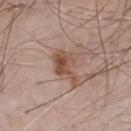Part of a total-body skin-imaging series; this lesion was reviewed on a skin check and was not flagged for biopsy. A region of skin cropped from a whole-body photographic capture, roughly 15 mm wide. Longest diameter approximately 4.5 mm. A male subject, aged 53–57. The total-body-photography lesion software estimated a footprint of about 7.5 mm², a shape eccentricity near 0.85, and two-axis asymmetry of about 0.45. It also reported a classifier nevus-likeness of about 65/100. The tile uses white-light illumination. The lesion is on the chest.